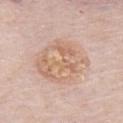{"biopsy_status": "not biopsied; imaged during a skin examination", "patient": {"sex": "male", "age_approx": 80}, "image": {"source": "total-body photography crop", "field_of_view_mm": 15}, "automated_metrics": {"area_mm2_approx": 30.0, "eccentricity": 0.35, "vs_skin_darker_L": 7.0, "vs_skin_contrast_norm": 6.0, "border_irregularity_0_10": 1.5, "color_variation_0_10": 6.5, "peripheral_color_asymmetry": 2.5, "nevus_likeness_0_100": 5, "lesion_detection_confidence_0_100": 100}, "lesion_size": {"long_diameter_mm_approx": 6.5}, "lighting": "white-light", "site": "chest"}